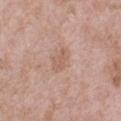Captured during whole-body skin photography for melanoma surveillance; the lesion was not biopsied.
Imaged with white-light lighting.
The patient is a male about 50 years old.
A roughly 15 mm field-of-view crop from a total-body skin photograph.
Located on the chest.
The lesion-visualizer software estimated a lesion area of about 5 mm², a shape eccentricity near 0.8, and a shape-asymmetry score of about 0.2 (0 = symmetric). The software also gave a classifier nevus-likeness of about 0/100 and lesion-presence confidence of about 100/100.